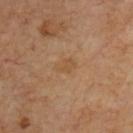Impression:
No biopsy was performed on this lesion — it was imaged during a full skin examination and was not determined to be concerning.
Clinical summary:
A male subject aged 63 to 67. Cropped from a whole-body photographic skin survey; the tile spans about 15 mm.